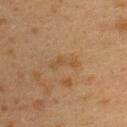Assessment: This lesion was catalogued during total-body skin photography and was not selected for biopsy. Context: This is a cross-polarized tile. About 3.5 mm across. A lesion tile, about 15 mm wide, cut from a 3D total-body photograph. From the upper back. A female subject, aged 38–42.